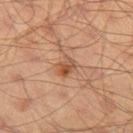  biopsy_status: not biopsied; imaged during a skin examination
  patient:
    sex: male
    age_approx: 55
  image:
    source: total-body photography crop
    field_of_view_mm: 15
  site: left thigh